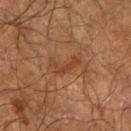Part of a total-body skin-imaging series; this lesion was reviewed on a skin check and was not flagged for biopsy. The lesion is on the left forearm. An algorithmic analysis of the crop reported an eccentricity of roughly 0.9 and a shape-asymmetry score of about 0.75 (0 = symmetric). The analysis additionally found a lesion color around L≈34 a*≈20 b*≈29 in CIELAB, about 6 CIELAB-L* units darker than the surrounding skin, and a normalized lesion–skin contrast near 5.5. It also reported a border-irregularity rating of about 8.5/10, a within-lesion color-variation index near 0/10, and peripheral color asymmetry of about 0. It also reported a detector confidence of about 95 out of 100 that the crop contains a lesion. The tile uses cross-polarized illumination. A roughly 15 mm field-of-view crop from a total-body skin photograph. A male subject roughly 70 years of age.